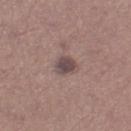Clinical impression: No biopsy was performed on this lesion — it was imaged during a full skin examination and was not determined to be concerning. Clinical summary: Imaged with white-light lighting. This image is a 15 mm lesion crop taken from a total-body photograph. The total-body-photography lesion software estimated a lesion area of about 5.5 mm², an outline eccentricity of about 0.65 (0 = round, 1 = elongated), and two-axis asymmetry of about 0.25. And it measured a mean CIELAB color near L≈47 a*≈15 b*≈17 and a normalized border contrast of about 8.5. It also reported a border-irregularity rating of about 2/10 and peripheral color asymmetry of about 1. The analysis additionally found a classifier nevus-likeness of about 35/100 and a detector confidence of about 100 out of 100 that the crop contains a lesion. A female subject, in their 20s. The lesion is located on the left thigh.